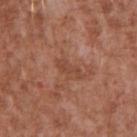Q: Was a biopsy performed?
A: catalogued during a skin exam; not biopsied
Q: What is the imaging modality?
A: 15 mm crop, total-body photography
Q: How large is the lesion?
A: about 3.5 mm
Q: What lighting was used for the tile?
A: white-light illumination
Q: Lesion location?
A: the chest
Q: What are the patient's age and sex?
A: male, aged 43–47